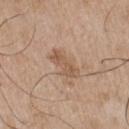{"biopsy_status": "not biopsied; imaged during a skin examination", "patient": {"sex": "male", "age_approx": 65}, "automated_metrics": {"eccentricity": 0.9, "shape_asymmetry": 0.25, "cielab_L": 55, "cielab_a": 18, "cielab_b": 31, "vs_skin_contrast_norm": 6.5, "nevus_likeness_0_100": 10, "lesion_detection_confidence_0_100": 100}, "lesion_size": {"long_diameter_mm_approx": 4.0}, "image": {"source": "total-body photography crop", "field_of_view_mm": 15}, "site": "chest", "lighting": "white-light"}